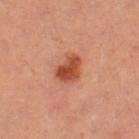lighting = cross-polarized; imaging modality = total-body-photography crop, ~15 mm field of view; subject = female, in their mid- to late 30s; anatomic site = the leg; size = about 3.5 mm.